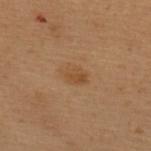The lesion is on the upper back. A female patient aged 48–52. A lesion tile, about 15 mm wide, cut from a 3D total-body photograph.